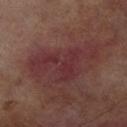biopsy_status: not biopsied; imaged during a skin examination
site: right lower leg
image:
  source: total-body photography crop
  field_of_view_mm: 15
lesion_size:
  long_diameter_mm_approx: 8.0
patient:
  sex: male
  age_approx: 70
lighting: cross-polarized